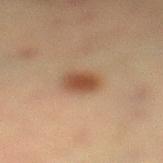Imaged during a routine full-body skin examination; the lesion was not biopsied and no histopathology is available. A 15 mm close-up extracted from a 3D total-body photography capture. From the left lower leg. A female patient, about 55 years old.